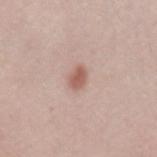{"patient": {"sex": "female", "age_approx": 50}, "site": "left forearm", "image": {"source": "total-body photography crop", "field_of_view_mm": 15}, "lesion_size": {"long_diameter_mm_approx": 2.5}, "lighting": "white-light"}